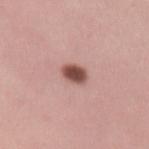Assessment:
Recorded during total-body skin imaging; not selected for excision or biopsy.
Clinical summary:
A female patient aged approximately 25. A 15 mm crop from a total-body photograph taken for skin-cancer surveillance. The lesion is on the left forearm. Imaged with white-light lighting. Automated tile analysis of the lesion measured a lesion color around L≈50 a*≈23 b*≈23 in CIELAB and a normalized border contrast of about 11.5. It also reported a border-irregularity rating of about 1.5/10, a color-variation rating of about 3/10, and radial color variation of about 1. The software also gave a nevus-likeness score of about 100/100.